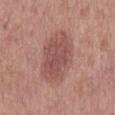Clinical summary: Automated image analysis of the tile measured an area of roughly 23 mm², an outline eccentricity of about 0.85 (0 = round, 1 = elongated), and a shape-asymmetry score of about 0.1 (0 = symmetric). It also reported a border-irregularity index near 2/10, internal color variation of about 3 on a 0–10 scale, and peripheral color asymmetry of about 1. The analysis additionally found an automated nevus-likeness rating near 55 out of 100 and a detector confidence of about 100 out of 100 that the crop contains a lesion. The tile uses white-light illumination. A 15 mm close-up tile from a total-body photography series done for melanoma screening. A male patient, in their 60s. Located on the mid back.